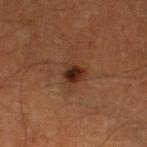workup: total-body-photography surveillance lesion; no biopsy | automated lesion analysis: an area of roughly 4.5 mm² and an outline eccentricity of about 0.55 (0 = round, 1 = elongated); a nevus-likeness score of about 95/100 and a lesion-detection confidence of about 100/100 | imaging modality: ~15 mm crop, total-body skin-cancer survey | lighting: cross-polarized illumination | lesion size: ~2.5 mm (longest diameter) | subject: male, aged approximately 65 | body site: the left lower leg.